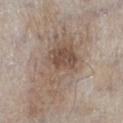workup — no biopsy performed (imaged during a skin exam)
anatomic site — the left lower leg
lighting — white-light
patient — male, aged 58–62
imaging modality — total-body-photography crop, ~15 mm field of view
lesion diameter — about 7.5 mm
automated lesion analysis — a lesion area of about 23 mm²; a border-irregularity rating of about 5/10, a color-variation rating of about 6.5/10, and peripheral color asymmetry of about 2; a nevus-likeness score of about 25/100 and lesion-presence confidence of about 100/100